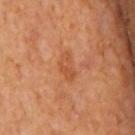• biopsy status · catalogued during a skin exam; not biopsied
• acquisition · ~15 mm tile from a whole-body skin photo
• patient · male, aged around 65
• diameter · ≈3.5 mm
• anatomic site · the mid back